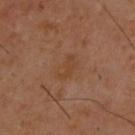Clinical impression: Part of a total-body skin-imaging series; this lesion was reviewed on a skin check and was not flagged for biopsy. Background: A male subject, in their 40s. Imaged with cross-polarized lighting. The lesion is on the upper back. A roughly 15 mm field-of-view crop from a total-body skin photograph.